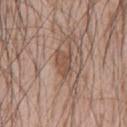notes — imaged on a skin check; not biopsied | anatomic site — the chest | lesion diameter — ~3.5 mm (longest diameter) | subject — male, approximately 55 years of age | lighting — white-light | acquisition — ~15 mm tile from a whole-body skin photo.